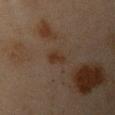{
  "patient": {
    "sex": "female",
    "age_approx": 45
  },
  "site": "left upper arm",
  "image": {
    "source": "total-body photography crop",
    "field_of_view_mm": 15
  },
  "lighting": "cross-polarized",
  "lesion_size": {
    "long_diameter_mm_approx": 3.0
  }
}